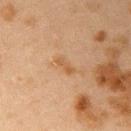{
  "biopsy_status": "not biopsied; imaged during a skin examination",
  "lighting": "cross-polarized",
  "patient": {
    "sex": "female",
    "age_approx": 40
  },
  "site": "right upper arm",
  "automated_metrics": {
    "area_mm2_approx": 2.5,
    "shape_asymmetry": 0.35,
    "cielab_L": 47,
    "cielab_a": 18,
    "cielab_b": 33,
    "vs_skin_darker_L": 6.0,
    "vs_skin_contrast_norm": 6.0,
    "border_irregularity_0_10": 4.0,
    "peripheral_color_asymmetry": 0.0
  },
  "image": {
    "source": "total-body photography crop",
    "field_of_view_mm": 15
  }
}